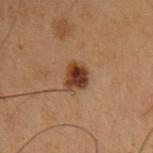Recorded during total-body skin imaging; not selected for excision or biopsy.
A male patient aged approximately 55.
Located on the right upper arm.
A 15 mm crop from a total-body photograph taken for skin-cancer surveillance.
This is a cross-polarized tile.
The lesion's longest dimension is about 3 mm.